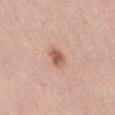biopsy status = no biopsy performed (imaged during a skin exam) | lesion diameter = ~2.5 mm (longest diameter) | image = 15 mm crop, total-body photography | lighting = white-light illumination | anatomic site = the leg | TBP lesion metrics = a lesion color around L≈59 a*≈22 b*≈31 in CIELAB; a border-irregularity index near 1.5/10, a color-variation rating of about 3/10, and a peripheral color-asymmetry measure near 1 | subject = male, aged 58–62.